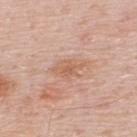No biopsy was performed on this lesion — it was imaged during a full skin examination and was not determined to be concerning.
On the upper back.
This is a white-light tile.
A lesion tile, about 15 mm wide, cut from a 3D total-body photograph.
Measured at roughly 4 mm in maximum diameter.
A male subject aged 48–52.
An algorithmic analysis of the crop reported a lesion area of about 6.5 mm², an eccentricity of roughly 0.8, and two-axis asymmetry of about 0.25. The analysis additionally found a mean CIELAB color near L≈61 a*≈21 b*≈31, roughly 8 lightness units darker than nearby skin, and a normalized lesion–skin contrast near 6. The analysis additionally found a nevus-likeness score of about 0/100 and a detector confidence of about 100 out of 100 that the crop contains a lesion.